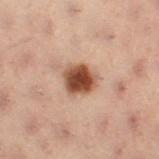<case>
<biopsy_status>not biopsied; imaged during a skin examination</biopsy_status>
<lighting>cross-polarized</lighting>
<image>
  <source>total-body photography crop</source>
  <field_of_view_mm>15</field_of_view_mm>
</image>
<lesion_size>
  <long_diameter_mm_approx>3.5</long_diameter_mm_approx>
</lesion_size>
<site>left leg</site>
<patient>
  <sex>female</sex>
  <age_approx>55</age_approx>
</patient>
</case>